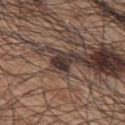workup: no biopsy performed (imaged during a skin exam)
patient: male, approximately 70 years of age
tile lighting: white-light illumination
image-analysis metrics: a mean CIELAB color near L≈33 a*≈13 b*≈18, roughly 13 lightness units darker than nearby skin, and a normalized lesion–skin contrast near 12.5; an automated nevus-likeness rating near 20 out of 100 and lesion-presence confidence of about 70/100
location: the upper back
image source: 15 mm crop, total-body photography
diameter: ≈2.5 mm